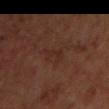Image and clinical context:
Captured under cross-polarized illumination. Longest diameter approximately 3.5 mm. A male patient, aged around 70. Cropped from a total-body skin-imaging series; the visible field is about 15 mm. The lesion-visualizer software estimated a mean CIELAB color near L≈25 a*≈19 b*≈23, a lesion–skin lightness drop of about 4, and a normalized border contrast of about 5. It also reported border irregularity of about 4.5 on a 0–10 scale, a within-lesion color-variation index near 0.5/10, and peripheral color asymmetry of about 0. It also reported a classifier nevus-likeness of about 0/100 and a detector confidence of about 100 out of 100 that the crop contains a lesion. On the chest.